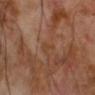The patient is a male aged approximately 70.
The recorded lesion diameter is about 2.5 mm.
The lesion is on the arm.
A 15 mm crop from a total-body photograph taken for skin-cancer surveillance.